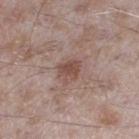<lesion>
<biopsy_status>not biopsied; imaged during a skin examination</biopsy_status>
<lighting>white-light</lighting>
<image>
  <source>total-body photography crop</source>
  <field_of_view_mm>15</field_of_view_mm>
</image>
<site>right thigh</site>
<automated_metrics>
  <area_mm2_approx>4.5</area_mm2_approx>
  <eccentricity>0.45</eccentricity>
  <shape_asymmetry>0.3</shape_asymmetry>
</automated_metrics>
<lesion_size>
  <long_diameter_mm_approx>2.5</long_diameter_mm_approx>
</lesion_size>
<patient>
  <sex>male</sex>
  <age_approx>45</age_approx>
</patient>
</lesion>